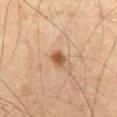Assessment: The lesion was photographed on a routine skin check and not biopsied; there is no pathology result. Clinical summary: A male patient, aged around 70. A roughly 15 mm field-of-view crop from a total-body skin photograph. From the abdomen. The total-body-photography lesion software estimated a footprint of about 3 mm² and an eccentricity of roughly 0.65. And it measured an average lesion color of about L≈53 a*≈22 b*≈36 (CIELAB), a lesion–skin lightness drop of about 13, and a lesion-to-skin contrast of about 9.5 (normalized; higher = more distinct). It also reported a color-variation rating of about 3.5/10 and a peripheral color-asymmetry measure near 1. The analysis additionally found an automated nevus-likeness rating near 95 out of 100. The tile uses cross-polarized illumination.